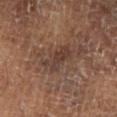This lesion was catalogued during total-body skin photography and was not selected for biopsy. A male patient in their mid- to late 60s. On the left lower leg. Imaged with cross-polarized lighting. About 4.5 mm across. A 15 mm close-up tile from a total-body photography series done for melanoma screening.